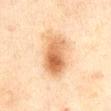<lesion>
  <biopsy_status>not biopsied; imaged during a skin examination</biopsy_status>
  <site>chest</site>
  <patient>
    <sex>male</sex>
    <age_approx>45</age_approx>
  </patient>
  <image>
    <source>total-body photography crop</source>
    <field_of_view_mm>15</field_of_view_mm>
  </image>
  <lighting>cross-polarized</lighting>
</lesion>